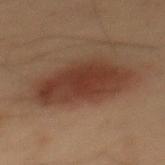Clinical impression: Recorded during total-body skin imaging; not selected for excision or biopsy. Acquisition and patient details: Captured under cross-polarized illumination. The subject is a male about 55 years old. This image is a 15 mm lesion crop taken from a total-body photograph. The recorded lesion diameter is about 8 mm. The lesion is on the mid back.